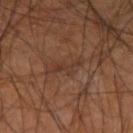biopsy status = catalogued during a skin exam; not biopsied
image = ~15 mm tile from a whole-body skin photo
site = the left forearm
automated metrics = a footprint of about 3.5 mm², an eccentricity of roughly 0.9, and two-axis asymmetry of about 0.65; an average lesion color of about L≈31 a*≈17 b*≈24 (CIELAB) and roughly 5 lightness units darker than nearby skin; border irregularity of about 8 on a 0–10 scale and a peripheral color-asymmetry measure near 0; an automated nevus-likeness rating near 0 out of 100 and a detector confidence of about 80 out of 100 that the crop contains a lesion
lighting = cross-polarized illumination
subject = male, aged approximately 60